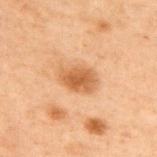Q: Is there a histopathology result?
A: no biopsy performed (imaged during a skin exam)
Q: What did automated image analysis measure?
A: a lesion area of about 8.5 mm², an outline eccentricity of about 0.75 (0 = round, 1 = elongated), and a shape-asymmetry score of about 0.2 (0 = symmetric); a lesion color around L≈46 a*≈19 b*≈33 in CIELAB; a classifier nevus-likeness of about 80/100 and a lesion-detection confidence of about 100/100
Q: Lesion location?
A: the upper back
Q: How was the tile lit?
A: cross-polarized illumination
Q: What is the imaging modality?
A: ~15 mm crop, total-body skin-cancer survey
Q: How large is the lesion?
A: ~4 mm (longest diameter)
Q: Who is the patient?
A: male, about 70 years old